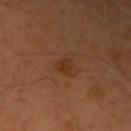Case summary:
• follow-up · catalogued during a skin exam; not biopsied
• site · the right upper arm
• imaging modality · 15 mm crop, total-body photography
• subject · male, aged 53–57
• lighting · cross-polarized illumination
• diameter · ~2 mm (longest diameter)